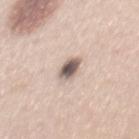Clinical impression:
This lesion was catalogued during total-body skin photography and was not selected for biopsy.
Clinical summary:
The lesion is located on the mid back. A lesion tile, about 15 mm wide, cut from a 3D total-body photograph. A male subject, in their mid- to late 40s. The tile uses white-light illumination.